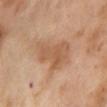Clinical impression: The lesion was photographed on a routine skin check and not biopsied; there is no pathology result. Acquisition and patient details: The lesion is on the abdomen. About 6 mm across. Imaged with cross-polarized lighting. Cropped from a total-body skin-imaging series; the visible field is about 15 mm. A female patient approximately 55 years of age.